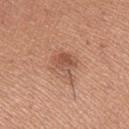Captured during whole-body skin photography for melanoma surveillance; the lesion was not biopsied.
A region of skin cropped from a whole-body photographic capture, roughly 15 mm wide.
The recorded lesion diameter is about 4 mm.
Automated image analysis of the tile measured an average lesion color of about L≈54 a*≈22 b*≈31 (CIELAB), a lesion–skin lightness drop of about 9, and a normalized lesion–skin contrast near 6.5.
The lesion is located on the left upper arm.
The subject is a female aged approximately 25.
Captured under white-light illumination.